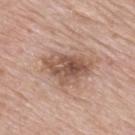Captured during whole-body skin photography for melanoma surveillance; the lesion was not biopsied. Captured under white-light illumination. A male subject, aged approximately 75. Cropped from a total-body skin-imaging series; the visible field is about 15 mm. An algorithmic analysis of the crop reported a lesion area of about 17 mm², an outline eccentricity of about 0.8 (0 = round, 1 = elongated), and a symmetry-axis asymmetry near 0.35. It also reported a mean CIELAB color near L≈54 a*≈19 b*≈27, about 12 CIELAB-L* units darker than the surrounding skin, and a lesion-to-skin contrast of about 8 (normalized; higher = more distinct). The analysis additionally found a border-irregularity index near 4/10, a color-variation rating of about 7/10, and peripheral color asymmetry of about 2.5. The analysis additionally found an automated nevus-likeness rating near 35 out of 100 and a lesion-detection confidence of about 100/100. Located on the mid back.